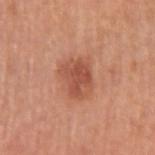Impression:
The lesion was tiled from a total-body skin photograph and was not biopsied.
Image and clinical context:
About 4 mm across. Located on the arm. This is a white-light tile. The patient is a male about 55 years old. Cropped from a whole-body photographic skin survey; the tile spans about 15 mm.